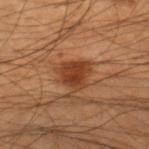Imaged during a routine full-body skin examination; the lesion was not biopsied and no histopathology is available. Captured under cross-polarized illumination. The lesion-visualizer software estimated an average lesion color of about L≈38 a*≈24 b*≈33 (CIELAB) and about 10 CIELAB-L* units darker than the surrounding skin. And it measured a border-irregularity rating of about 3.5/10 and internal color variation of about 3.5 on a 0–10 scale. And it measured a classifier nevus-likeness of about 90/100. A male subject in their mid- to late 40s. A roughly 15 mm field-of-view crop from a total-body skin photograph. The lesion is on the leg.